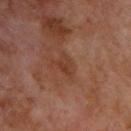The lesion was tiled from a total-body skin photograph and was not biopsied. Longest diameter approximately 2.5 mm. A male patient, aged 68 to 72. The lesion is on the back. Cropped from a total-body skin-imaging series; the visible field is about 15 mm. Imaged with cross-polarized lighting.